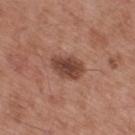Q: Was this lesion biopsied?
A: no biopsy performed (imaged during a skin exam)
Q: Who is the patient?
A: male, roughly 55 years of age
Q: What did automated image analysis measure?
A: a normalized lesion–skin contrast near 9.5; border irregularity of about 2 on a 0–10 scale and radial color variation of about 1
Q: What is the anatomic site?
A: the mid back
Q: What is the lesion's diameter?
A: about 4.5 mm
Q: What lighting was used for the tile?
A: white-light
Q: What is the imaging modality?
A: ~15 mm tile from a whole-body skin photo